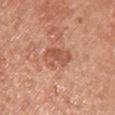Assessment:
The lesion was tiled from a total-body skin photograph and was not biopsied.
Clinical summary:
The lesion is on the chest. A female subject, aged around 65. A region of skin cropped from a whole-body photographic capture, roughly 15 mm wide.